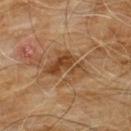notes = imaged on a skin check; not biopsied
image = total-body-photography crop, ~15 mm field of view
patient = male, roughly 60 years of age
location = the chest